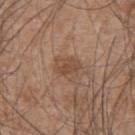The lesion was tiled from a total-body skin photograph and was not biopsied.
A region of skin cropped from a whole-body photographic capture, roughly 15 mm wide.
The lesion's longest dimension is about 3 mm.
The subject is a male aged around 45.
On the back.
Automated image analysis of the tile measured a lesion color around L≈48 a*≈18 b*≈29 in CIELAB, a lesion–skin lightness drop of about 8, and a lesion-to-skin contrast of about 6 (normalized; higher = more distinct). The analysis additionally found an automated nevus-likeness rating near 20 out of 100 and lesion-presence confidence of about 100/100.